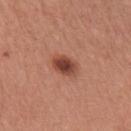The total-body-photography lesion software estimated a footprint of about 6 mm² and a symmetry-axis asymmetry near 0.2. The analysis additionally found roughly 14 lightness units darker than nearby skin. And it measured a nevus-likeness score of about 100/100. This is a white-light tile. The patient is a female roughly 65 years of age. Measured at roughly 3 mm in maximum diameter. A close-up tile cropped from a whole-body skin photograph, about 15 mm across. On the right upper arm.